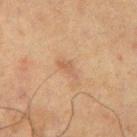<lesion>
<biopsy_status>not biopsied; imaged during a skin examination</biopsy_status>
<site>right upper arm</site>
<lesion_size>
  <long_diameter_mm_approx>2.5</long_diameter_mm_approx>
</lesion_size>
<lighting>cross-polarized</lighting>
<patient>
  <sex>male</sex>
  <age_approx>70</age_approx>
</patient>
<image>
  <source>total-body photography crop</source>
  <field_of_view_mm>15</field_of_view_mm>
</image>
</lesion>